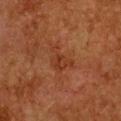Q: Was this lesion biopsied?
A: imaged on a skin check; not biopsied